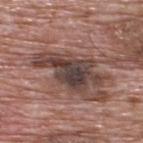biopsy_status: not biopsied; imaged during a skin examination
patient:
  sex: male
  age_approx: 70
lesion_size:
  long_diameter_mm_approx: 8.0
lighting: white-light
site: upper back
image:
  source: total-body photography crop
  field_of_view_mm: 15